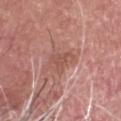lighting = white-light illumination | image source = total-body-photography crop, ~15 mm field of view | patient = male, approximately 55 years of age | diameter = ≈2.5 mm | image-analysis metrics = a mean CIELAB color near L≈52 a*≈24 b*≈27, a lesion–skin lightness drop of about 7, and a normalized lesion–skin contrast near 5.5 | anatomic site = the head or neck.